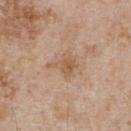Notes:
• biopsy status: catalogued during a skin exam; not biopsied
• lesion diameter: about 3.5 mm
• subject: male, aged approximately 65
• lighting: white-light
• TBP lesion metrics: a border-irregularity index near 4.5/10, internal color variation of about 3 on a 0–10 scale, and peripheral color asymmetry of about 1; a classifier nevus-likeness of about 0/100 and a detector confidence of about 100 out of 100 that the crop contains a lesion
• image source: ~15 mm crop, total-body skin-cancer survey
• body site: the chest